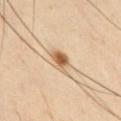• notes: total-body-photography surveillance lesion; no biopsy
• patient: male, in their mid- to late 40s
• location: the chest
• image: 15 mm crop, total-body photography
• diameter: about 3 mm